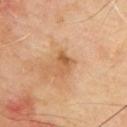The lesion was tiled from a total-body skin photograph and was not biopsied. Captured under cross-polarized illumination. The lesion is on the upper back. The recorded lesion diameter is about 3 mm. A male subject aged 63–67. The total-body-photography lesion software estimated a footprint of about 4 mm² and a shape eccentricity near 0.75. It also reported a nevus-likeness score of about 0/100 and lesion-presence confidence of about 100/100. A roughly 15 mm field-of-view crop from a total-body skin photograph.